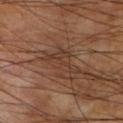Impression:
Part of a total-body skin-imaging series; this lesion was reviewed on a skin check and was not flagged for biopsy.
Image and clinical context:
Approximately 5 mm at its widest. The lesion is located on the left lower leg. A 15 mm crop from a total-body photograph taken for skin-cancer surveillance. This is a cross-polarized tile. Automated image analysis of the tile measured roughly 6 lightness units darker than nearby skin. And it measured internal color variation of about 2.5 on a 0–10 scale and radial color variation of about 1. A male patient about 60 years old.